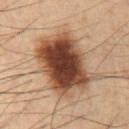Captured during whole-body skin photography for melanoma surveillance; the lesion was not biopsied.
A male patient aged approximately 60.
An algorithmic analysis of the crop reported an average lesion color of about L≈44 a*≈20 b*≈30 (CIELAB) and a normalized lesion–skin contrast near 13.5. It also reported a border-irregularity index near 2.5/10, internal color variation of about 8.5 on a 0–10 scale, and a peripheral color-asymmetry measure near 2.5.
Captured under cross-polarized illumination.
From the chest.
A 15 mm crop from a total-body photograph taken for skin-cancer surveillance.
The recorded lesion diameter is about 8 mm.